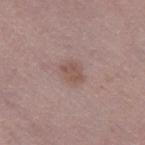Q: Is there a histopathology result?
A: no biopsy performed (imaged during a skin exam)
Q: What are the patient's age and sex?
A: female, aged around 50
Q: How was the tile lit?
A: white-light
Q: How large is the lesion?
A: about 2.5 mm
Q: What did automated image analysis measure?
A: a lesion area of about 4 mm², an eccentricity of roughly 0.7, and a symmetry-axis asymmetry near 0.25; an average lesion color of about L≈51 a*≈18 b*≈23 (CIELAB) and a lesion–skin lightness drop of about 8; an automated nevus-likeness rating near 30 out of 100 and a detector confidence of about 100 out of 100 that the crop contains a lesion
Q: Where on the body is the lesion?
A: the left thigh
Q: How was this image acquired?
A: total-body-photography crop, ~15 mm field of view